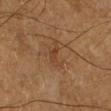{"image": {"source": "total-body photography crop", "field_of_view_mm": 15}, "lighting": "cross-polarized", "site": "leg", "patient": {"sex": "male", "age_approx": 55}}